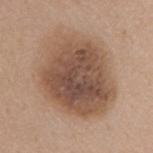| key | value |
|---|---|
| workup | no biopsy performed (imaged during a skin exam) |
| image | ~15 mm tile from a whole-body skin photo |
| anatomic site | the mid back |
| patient | female, in their mid-60s |